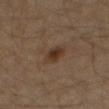Q: Was this lesion biopsied?
A: no biopsy performed (imaged during a skin exam)
Q: How was the tile lit?
A: cross-polarized
Q: Where on the body is the lesion?
A: the right thigh
Q: What kind of image is this?
A: ~15 mm crop, total-body skin-cancer survey
Q: Who is the patient?
A: male, aged 58 to 62